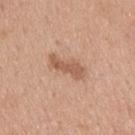biopsy status=imaged on a skin check; not biopsied | body site=the upper back | patient=male, aged 33 to 37 | acquisition=15 mm crop, total-body photography.